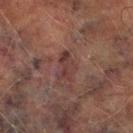Assessment:
This lesion was catalogued during total-body skin photography and was not selected for biopsy.